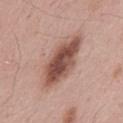Recorded during total-body skin imaging; not selected for excision or biopsy. A 15 mm close-up extracted from a 3D total-body photography capture. The lesion is located on the mid back. The patient is a male aged approximately 55. Imaged with white-light lighting. The recorded lesion diameter is about 7.5 mm.